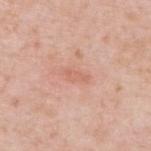Impression:
The lesion was tiled from a total-body skin photograph and was not biopsied.
Image and clinical context:
On the upper back. The patient is a male about 50 years old. This image is a 15 mm lesion crop taken from a total-body photograph. About 3.5 mm across. An algorithmic analysis of the crop reported an outline eccentricity of about 0.9 (0 = round, 1 = elongated). The analysis additionally found an average lesion color of about L≈64 a*≈24 b*≈30 (CIELAB), about 7 CIELAB-L* units darker than the surrounding skin, and a lesion-to-skin contrast of about 4.5 (normalized; higher = more distinct). The software also gave border irregularity of about 4 on a 0–10 scale and peripheral color asymmetry of about 0.